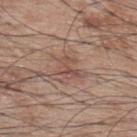Assessment: The lesion was tiled from a total-body skin photograph and was not biopsied. Clinical summary: The patient is a male roughly 70 years of age. A close-up tile cropped from a whole-body skin photograph, about 15 mm across. The lesion is located on the upper back.